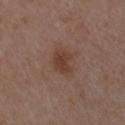Captured during whole-body skin photography for melanoma surveillance; the lesion was not biopsied. Imaged with white-light lighting. A region of skin cropped from a whole-body photographic capture, roughly 15 mm wide. The recorded lesion diameter is about 3 mm. The lesion is located on the arm. A male subject in their 50s.